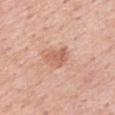follow-up=catalogued during a skin exam; not biopsied
image=~15 mm tile from a whole-body skin photo
subject=male, in their mid- to late 50s
location=the right upper arm
lesion diameter=≈3.5 mm
lighting=white-light